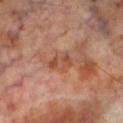Case summary:
* biopsy status: total-body-photography surveillance lesion; no biopsy
* TBP lesion metrics: a mean CIELAB color near L≈48 a*≈25 b*≈31
* subject: male, aged around 70
* tile lighting: cross-polarized
* body site: the leg
* diameter: ≈3 mm
* imaging modality: ~15 mm crop, total-body skin-cancer survey